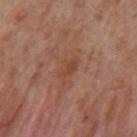The lesion was photographed on a routine skin check and not biopsied; there is no pathology result. The tile uses cross-polarized illumination. About 3 mm across. A female subject in their 50s. This image is a 15 mm lesion crop taken from a total-body photograph. On the right upper arm. The lesion-visualizer software estimated an area of roughly 4 mm², a shape eccentricity near 0.85, and a symmetry-axis asymmetry near 0.3. The analysis additionally found an average lesion color of about L≈42 a*≈21 b*≈31 (CIELAB), roughly 6 lightness units darker than nearby skin, and a normalized border contrast of about 6. And it measured border irregularity of about 3.5 on a 0–10 scale, a within-lesion color-variation index near 1/10, and peripheral color asymmetry of about 0.5.